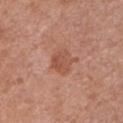Q: Was this lesion biopsied?
A: no biopsy performed (imaged during a skin exam)
Q: What are the patient's age and sex?
A: female, aged 48 to 52
Q: Where on the body is the lesion?
A: the chest
Q: What lighting was used for the tile?
A: white-light
Q: What is the imaging modality?
A: ~15 mm crop, total-body skin-cancer survey
Q: Lesion size?
A: about 3 mm
Q: Automated lesion metrics?
A: a lesion–skin lightness drop of about 8; border irregularity of about 2 on a 0–10 scale and internal color variation of about 3 on a 0–10 scale